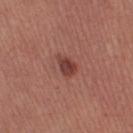follow-up: total-body-photography surveillance lesion; no biopsy
size: ≈3 mm
site: the right thigh
TBP lesion metrics: a lesion color around L≈40 a*≈26 b*≈24 in CIELAB and roughly 11 lightness units darker than nearby skin; an automated nevus-likeness rating near 90 out of 100
acquisition: total-body-photography crop, ~15 mm field of view
patient: female, aged around 30